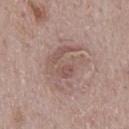Assessment: The lesion was tiled from a total-body skin photograph and was not biopsied. Context: A male patient aged approximately 70. The tile uses white-light illumination. A 15 mm close-up extracted from a 3D total-body photography capture. From the mid back. Longest diameter approximately 6 mm. An algorithmic analysis of the crop reported an area of roughly 16 mm², an outline eccentricity of about 0.7 (0 = round, 1 = elongated), and two-axis asymmetry of about 0.35.